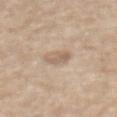This lesion was catalogued during total-body skin photography and was not selected for biopsy.